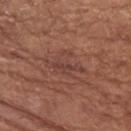Impression: The lesion was photographed on a routine skin check and not biopsied; there is no pathology result. Background: A 15 mm close-up extracted from a 3D total-body photography capture. A female subject, in their mid- to late 70s. From the left upper arm.